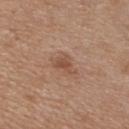{"biopsy_status": "not biopsied; imaged during a skin examination", "lesion_size": {"long_diameter_mm_approx": 3.0}, "patient": {"sex": "male", "age_approx": 65}, "image": {"source": "total-body photography crop", "field_of_view_mm": 15}, "site": "chest", "automated_metrics": {"eccentricity": 0.8, "shape_asymmetry": 0.4, "cielab_L": 50, "cielab_a": 20, "cielab_b": 29, "vs_skin_darker_L": 8.0, "vs_skin_contrast_norm": 6.5, "border_irregularity_0_10": 4.0, "color_variation_0_10": 2.0, "peripheral_color_asymmetry": 0.5, "nevus_likeness_0_100": 10, "lesion_detection_confidence_0_100": 100}, "lighting": "white-light"}